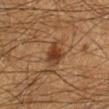A 15 mm close-up extracted from a 3D total-body photography capture. The lesion is on the left lower leg. A male patient, roughly 65 years of age.